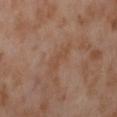Recorded during total-body skin imaging; not selected for excision or biopsy.